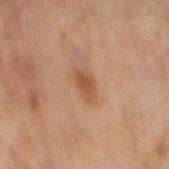Impression:
This lesion was catalogued during total-body skin photography and was not selected for biopsy.
Acquisition and patient details:
A close-up tile cropped from a whole-body skin photograph, about 15 mm across. The lesion is on the mid back. A male subject approximately 65 years of age. Imaged with cross-polarized lighting.